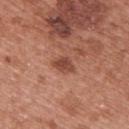| field | value |
|---|---|
| biopsy status | imaged on a skin check; not biopsied |
| lesion diameter | about 2.5 mm |
| imaging modality | total-body-photography crop, ~15 mm field of view |
| subject | female, roughly 40 years of age |
| illumination | white-light |
| site | the upper back |